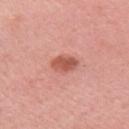Q: Was a biopsy performed?
A: no biopsy performed (imaged during a skin exam)
Q: Where on the body is the lesion?
A: the right upper arm
Q: Illumination type?
A: white-light
Q: What are the patient's age and sex?
A: female, aged around 50
Q: What is the imaging modality?
A: total-body-photography crop, ~15 mm field of view
Q: Automated lesion metrics?
A: an automated nevus-likeness rating near 90 out of 100 and a lesion-detection confidence of about 100/100